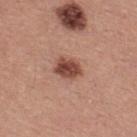| feature | finding |
|---|---|
| workup | imaged on a skin check; not biopsied |
| location | the upper back |
| patient | male, about 40 years old |
| acquisition | total-body-photography crop, ~15 mm field of view |
| lighting | white-light illumination |
| lesion size | ~3.5 mm (longest diameter) |